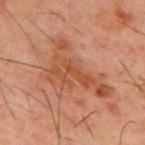The lesion was tiled from a total-body skin photograph and was not biopsied.